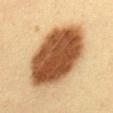Q: Is there a histopathology result?
A: total-body-photography surveillance lesion; no biopsy
Q: What is the lesion's diameter?
A: about 10 mm
Q: Patient demographics?
A: female, roughly 30 years of age
Q: Where on the body is the lesion?
A: the back
Q: How was this image acquired?
A: total-body-photography crop, ~15 mm field of view
Q: What lighting was used for the tile?
A: cross-polarized illumination